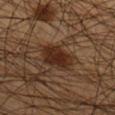The lesion was photographed on a routine skin check and not biopsied; there is no pathology result.
A male subject, aged 43–47.
Located on the right lower leg.
A 15 mm crop from a total-body photograph taken for skin-cancer surveillance.
The total-body-photography lesion software estimated a mean CIELAB color near L≈26 a*≈17 b*≈26 and about 10 CIELAB-L* units darker than the surrounding skin. And it measured an automated nevus-likeness rating near 85 out of 100 and lesion-presence confidence of about 100/100.